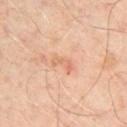- biopsy status: no biopsy performed (imaged during a skin exam)
- subject: male, aged around 50
- imaging modality: 15 mm crop, total-body photography
- site: the front of the torso